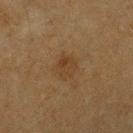biopsy status=no biopsy performed (imaged during a skin exam); site=the left upper arm; image=total-body-photography crop, ~15 mm field of view; subject=female, in their mid-50s; lesion size=≈2.5 mm.